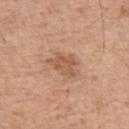Q: Is there a histopathology result?
A: catalogued during a skin exam; not biopsied
Q: Patient demographics?
A: male, aged approximately 55
Q: What is the imaging modality?
A: 15 mm crop, total-body photography
Q: What is the anatomic site?
A: the upper back